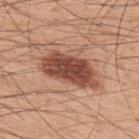Q: Is there a histopathology result?
A: total-body-photography surveillance lesion; no biopsy
Q: How large is the lesion?
A: about 7 mm
Q: Illumination type?
A: white-light
Q: What is the anatomic site?
A: the back
Q: What kind of image is this?
A: 15 mm crop, total-body photography
Q: Patient demographics?
A: male, about 60 years old